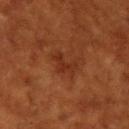  biopsy_status: not biopsied; imaged during a skin examination
  image:
    source: total-body photography crop
    field_of_view_mm: 15
  automated_metrics:
    area_mm2_approx: 4.5
    eccentricity: 0.8
    vs_skin_darker_L: 5.0
    border_irregularity_0_10: 5.5
    color_variation_0_10: 1.5
    nevus_likeness_0_100: 0
    lesion_detection_confidence_0_100: 100
  site: upper back
  patient:
    sex: female
    age_approx: 80